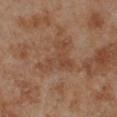| feature | finding |
|---|---|
| follow-up | imaged on a skin check; not biopsied |
| automated lesion analysis | a lesion color around L≈43 a*≈19 b*≈29 in CIELAB and a normalized lesion–skin contrast near 5 |
| body site | the left lower leg |
| patient | male, aged 68 to 72 |
| imaging modality | ~15 mm crop, total-body skin-cancer survey |
| lesion size | about 5 mm |